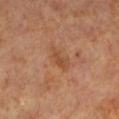Clinical impression: No biopsy was performed on this lesion — it was imaged during a full skin examination and was not determined to be concerning. Acquisition and patient details: A lesion tile, about 15 mm wide, cut from a 3D total-body photograph. Located on the left lower leg. A female patient, in their mid- to late 50s.